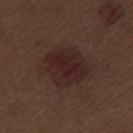| key | value |
|---|---|
| follow-up | catalogued during a skin exam; not biopsied |
| acquisition | ~15 mm tile from a whole-body skin photo |
| body site | the left thigh |
| subject | male, about 70 years old |